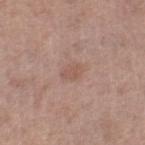{
  "biopsy_status": "not biopsied; imaged during a skin examination",
  "patient": {
    "sex": "female",
    "age_approx": 75
  },
  "image": {
    "source": "total-body photography crop",
    "field_of_view_mm": 15
  },
  "lesion_size": {
    "long_diameter_mm_approx": 2.5
  },
  "lighting": "white-light",
  "site": "right thigh"
}